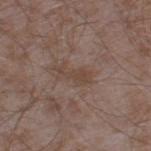Notes:
– biopsy status · total-body-photography surveillance lesion; no biopsy
– subject · male, in their mid-40s
– acquisition · total-body-photography crop, ~15 mm field of view
– body site · the left thigh